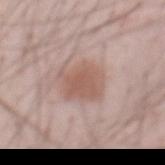Captured during whole-body skin photography for melanoma surveillance; the lesion was not biopsied. This is a white-light tile. Located on the abdomen. The patient is a male in their mid- to late 50s. The recorded lesion diameter is about 4 mm. The lesion-visualizer software estimated a footprint of about 13 mm², an eccentricity of roughly 0.4, and a shape-asymmetry score of about 0.2 (0 = symmetric). The software also gave border irregularity of about 2.5 on a 0–10 scale and a color-variation rating of about 2/10. It also reported a lesion-detection confidence of about 100/100. A 15 mm crop from a total-body photograph taken for skin-cancer surveillance.